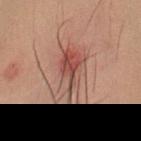{"biopsy_status": "not biopsied; imaged during a skin examination", "lesion_size": {"long_diameter_mm_approx": 7.0}, "image": {"source": "total-body photography crop", "field_of_view_mm": 15}, "site": "head or neck", "lighting": "cross-polarized", "patient": {"sex": "female", "age_approx": 20}}